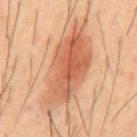biopsy_status: not biopsied; imaged during a skin examination
patient:
  sex: male
  age_approx: 40
automated_metrics:
  area_mm2_approx: 34.0
  eccentricity: 0.9
  shape_asymmetry: 0.25
  nevus_likeness_0_100: 100
site: mid back
image:
  source: total-body photography crop
  field_of_view_mm: 15